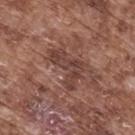<tbp_lesion>
<biopsy_status>not biopsied; imaged during a skin examination</biopsy_status>
<image>
  <source>total-body photography crop</source>
  <field_of_view_mm>15</field_of_view_mm>
</image>
<patient>
  <sex>male</sex>
  <age_approx>75</age_approx>
</patient>
<automated_metrics>
  <area_mm2_approx>10.0</area_mm2_approx>
  <shape_asymmetry>0.55</shape_asymmetry>
  <color_variation_0_10>4.0</color_variation_0_10>
  <peripheral_color_asymmetry>1.5</peripheral_color_asymmetry>
</automated_metrics>
<site>upper back</site>
</tbp_lesion>